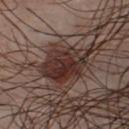<tbp_lesion>
  <biopsy_status>not biopsied; imaged during a skin examination</biopsy_status>
  <automated_metrics>
    <cielab_L>31</cielab_L>
    <cielab_a>18</cielab_a>
    <cielab_b>20</cielab_b>
    <vs_skin_darker_L>12.0</vs_skin_darker_L>
    <vs_skin_contrast_norm>11.0</vs_skin_contrast_norm>
    <border_irregularity_0_10>3.5</border_irregularity_0_10>
    <color_variation_0_10>5.5</color_variation_0_10>
    <peripheral_color_asymmetry>2.0</peripheral_color_asymmetry>
    <nevus_likeness_0_100>100</nevus_likeness_0_100>
    <lesion_detection_confidence_0_100>100</lesion_detection_confidence_0_100>
  </automated_metrics>
  <patient>
    <sex>male</sex>
    <age_approx>35</age_approx>
  </patient>
  <image>
    <source>total-body photography crop</source>
    <field_of_view_mm>15</field_of_view_mm>
  </image>
  <lesion_size>
    <long_diameter_mm_approx>6.0</long_diameter_mm_approx>
  </lesion_size>
  <site>chest</site>
  <lighting>white-light</lighting>
</tbp_lesion>